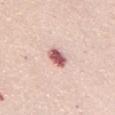The lesion was tiled from a total-body skin photograph and was not biopsied. From the back. The patient is a female roughly 45 years of age. A close-up tile cropped from a whole-body skin photograph, about 15 mm across. The tile uses white-light illumination.